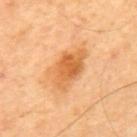<record>
  <biopsy_status>not biopsied; imaged during a skin examination</biopsy_status>
  <lighting>cross-polarized</lighting>
  <lesion_size>
    <long_diameter_mm_approx>5.0</long_diameter_mm_approx>
  </lesion_size>
  <patient>
    <sex>male</sex>
    <age_approx>65</age_approx>
  </patient>
  <site>mid back</site>
  <image>
    <source>total-body photography crop</source>
    <field_of_view_mm>15</field_of_view_mm>
  </image>
</record>